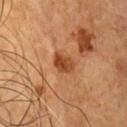imaging modality: ~15 mm crop, total-body skin-cancer survey | anatomic site: the chest | subject: male, approximately 55 years of age.